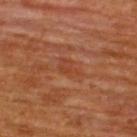notes = imaged on a skin check; not biopsied | acquisition = ~15 mm tile from a whole-body skin photo | site = the upper back | lesion size = ≈3.5 mm | automated metrics = border irregularity of about 3.5 on a 0–10 scale and radial color variation of about 1; a nevus-likeness score of about 0/100 | subject = male, aged around 65.